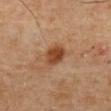The lesion was tiled from a total-body skin photograph and was not biopsied. The lesion-visualizer software estimated a shape eccentricity near 0.5 and a symmetry-axis asymmetry near 0.15. And it measured a border-irregularity rating of about 1.5/10 and a peripheral color-asymmetry measure near 1. Cropped from a whole-body photographic skin survey; the tile spans about 15 mm. On the abdomen. The recorded lesion diameter is about 3 mm. This is a cross-polarized tile. A male patient aged around 75.